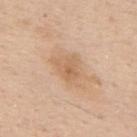* biopsy status: imaged on a skin check; not biopsied
* subject: male, aged approximately 55
* image source: ~15 mm tile from a whole-body skin photo
* automated lesion analysis: a lesion color around L≈63 a*≈18 b*≈35 in CIELAB; border irregularity of about 3 on a 0–10 scale, internal color variation of about 4 on a 0–10 scale, and peripheral color asymmetry of about 1
* diameter: about 3.5 mm
* lighting: white-light
* location: the upper back